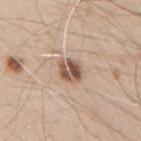Clinical impression:
The lesion was photographed on a routine skin check and not biopsied; there is no pathology result.
Clinical summary:
The lesion is on the arm. Cropped from a whole-body photographic skin survey; the tile spans about 15 mm. A male patient roughly 70 years of age.